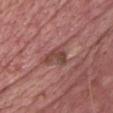biopsy_status: not biopsied; imaged during a skin examination
patient:
  sex: male
  age_approx: 60
image:
  source: total-body photography crop
  field_of_view_mm: 15
site: chest
lesion_size:
  long_diameter_mm_approx: 3.0
lighting: white-light
automated_metrics:
  border_irregularity_0_10: 4.5
  color_variation_0_10: 4.0
  peripheral_color_asymmetry: 1.5
  nevus_likeness_0_100: 0
  lesion_detection_confidence_0_100: 100